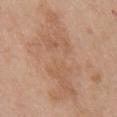biopsy status — imaged on a skin check; not biopsied | image source — ~15 mm tile from a whole-body skin photo | patient — female, in their 60s | body site — the front of the torso.